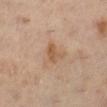Imaged during a routine full-body skin examination; the lesion was not biopsied and no histopathology is available.
The recorded lesion diameter is about 2.5 mm.
A region of skin cropped from a whole-body photographic capture, roughly 15 mm wide.
The lesion is located on the left lower leg.
Imaged with cross-polarized lighting.
The lesion-visualizer software estimated a border-irregularity rating of about 3.5/10, a within-lesion color-variation index near 1.5/10, and radial color variation of about 0.5.
The patient is a female about 50 years old.